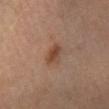Q: Is there a histopathology result?
A: imaged on a skin check; not biopsied
Q: Where on the body is the lesion?
A: the left lower leg
Q: What kind of image is this?
A: ~15 mm crop, total-body skin-cancer survey
Q: What are the patient's age and sex?
A: female, aged around 55